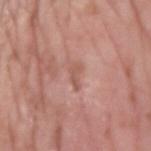biopsy status: catalogued during a skin exam; not biopsied | automated lesion analysis: a footprint of about 2.5 mm², an outline eccentricity of about 0.9 (0 = round, 1 = elongated), and a symmetry-axis asymmetry near 0.5; a mean CIELAB color near L≈55 a*≈24 b*≈27 and roughly 8 lightness units darker than nearby skin; a border-irregularity index near 5.5/10 and peripheral color asymmetry of about 0 | subject: male, aged 53–57 | site: the right upper arm | diameter: ~2.5 mm (longest diameter) | imaging modality: total-body-photography crop, ~15 mm field of view.